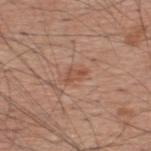Part of a total-body skin-imaging series; this lesion was reviewed on a skin check and was not flagged for biopsy. The lesion is located on the upper back. The tile uses white-light illumination. Cropped from a total-body skin-imaging series; the visible field is about 15 mm. The lesion-visualizer software estimated a lesion area of about 3 mm², an eccentricity of roughly 0.85, and a symmetry-axis asymmetry near 0.3. A male subject aged approximately 60.